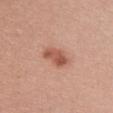Q: Was a biopsy performed?
A: total-body-photography surveillance lesion; no biopsy
Q: How was this image acquired?
A: 15 mm crop, total-body photography
Q: Illumination type?
A: white-light
Q: Automated lesion metrics?
A: an eccentricity of roughly 0.85 and a shape-asymmetry score of about 0.25 (0 = symmetric); a lesion color around L≈54 a*≈26 b*≈30 in CIELAB, a lesion–skin lightness drop of about 12, and a normalized border contrast of about 8; a border-irregularity index near 2.5/10, a within-lesion color-variation index near 3.5/10, and radial color variation of about 1.5
Q: Where on the body is the lesion?
A: the upper back
Q: Who is the patient?
A: female, about 20 years old